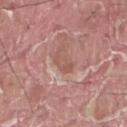Imaged during a routine full-body skin examination; the lesion was not biopsied and no histopathology is available.